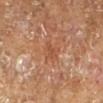Clinical impression:
The lesion was tiled from a total-body skin photograph and was not biopsied.
Clinical summary:
The lesion is on the right lower leg. This is a cross-polarized tile. Approximately 3 mm at its widest. A close-up tile cropped from a whole-body skin photograph, about 15 mm across. An algorithmic analysis of the crop reported a shape eccentricity near 0.65 and a shape-asymmetry score of about 0.35 (0 = symmetric). And it measured a color-variation rating of about 1.5/10. A male subject, aged 73–77.